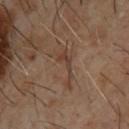{
  "biopsy_status": "not biopsied; imaged during a skin examination",
  "lesion_size": {
    "long_diameter_mm_approx": 5.0
  },
  "image": {
    "source": "total-body photography crop",
    "field_of_view_mm": 15
  },
  "lighting": "cross-polarized",
  "site": "upper back",
  "patient": {
    "sex": "male",
    "age_approx": 65
  }
}